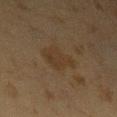- follow-up: imaged on a skin check; not biopsied
- location: the left upper arm
- illumination: cross-polarized illumination
- diameter: ≈4.5 mm
- patient: female, aged around 40
- acquisition: 15 mm crop, total-body photography